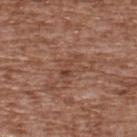- follow-up — imaged on a skin check; not biopsied